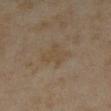Case summary:
– notes · imaged on a skin check; not biopsied
– size · ≈3.5 mm
– site · the left forearm
– patient · female, aged 33–37
– image · 15 mm crop, total-body photography
– lighting · cross-polarized